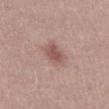This lesion was catalogued during total-body skin photography and was not selected for biopsy. The lesion-visualizer software estimated a mean CIELAB color near L≈54 a*≈21 b*≈22, about 10 CIELAB-L* units darker than the surrounding skin, and a lesion-to-skin contrast of about 7 (normalized; higher = more distinct). The analysis additionally found a within-lesion color-variation index near 2/10. A 15 mm close-up tile from a total-body photography series done for melanoma screening. The tile uses white-light illumination. The subject is a male aged 28 to 32. Measured at roughly 3.5 mm in maximum diameter.